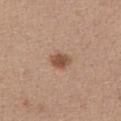follow-up — catalogued during a skin exam; not biopsied | tile lighting — white-light | TBP lesion metrics — an average lesion color of about L≈51 a*≈20 b*≈30 (CIELAB), roughly 12 lightness units darker than nearby skin, and a lesion-to-skin contrast of about 8.5 (normalized; higher = more distinct); an automated nevus-likeness rating near 95 out of 100 and lesion-presence confidence of about 100/100 | subject — female, aged approximately 35 | image — ~15 mm tile from a whole-body skin photo | diameter — ~2.5 mm (longest diameter) | anatomic site — the abdomen.